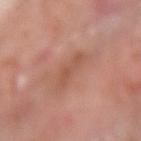Q: Is there a histopathology result?
A: no biopsy performed (imaged during a skin exam)
Q: What kind of image is this?
A: total-body-photography crop, ~15 mm field of view
Q: What is the anatomic site?
A: the right forearm
Q: Patient demographics?
A: male, about 75 years old